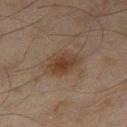Q: Is there a histopathology result?
A: imaged on a skin check; not biopsied
Q: Who is the patient?
A: male, aged approximately 45
Q: What did automated image analysis measure?
A: a footprint of about 9.5 mm², a shape eccentricity near 0.8, and a shape-asymmetry score of about 0.15 (0 = symmetric); a border-irregularity rating of about 2/10, a color-variation rating of about 3.5/10, and radial color variation of about 1
Q: What kind of image is this?
A: ~15 mm crop, total-body skin-cancer survey
Q: Lesion location?
A: the right thigh
Q: Lesion size?
A: ~5 mm (longest diameter)